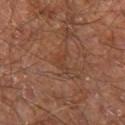Context:
The subject is a male aged around 60. On the right leg. About 2.5 mm across. A 15 mm close-up extracted from a 3D total-body photography capture. Captured under cross-polarized illumination.